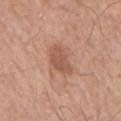Part of a total-body skin-imaging series; this lesion was reviewed on a skin check and was not flagged for biopsy. The tile uses white-light illumination. A 15 mm close-up extracted from a 3D total-body photography capture. Longest diameter approximately 3.5 mm. From the right upper arm. A male patient, about 55 years old.